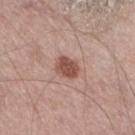The lesion was photographed on a routine skin check and not biopsied; there is no pathology result.
A male subject aged around 75.
Captured under white-light illumination.
Cropped from a total-body skin-imaging series; the visible field is about 15 mm.
The lesion-visualizer software estimated an area of roughly 5.5 mm², an outline eccentricity of about 0.5 (0 = round, 1 = elongated), and two-axis asymmetry of about 0.15. And it measured peripheral color asymmetry of about 1. The analysis additionally found a nevus-likeness score of about 80/100 and a detector confidence of about 100 out of 100 that the crop contains a lesion.
Located on the right thigh.
About 2.5 mm across.